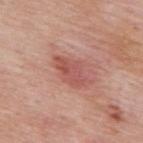Context: A lesion tile, about 15 mm wide, cut from a 3D total-body photograph. A male subject, in their mid- to late 50s. The lesion is located on the mid back. This is a white-light tile. Measured at roughly 4.5 mm in maximum diameter.